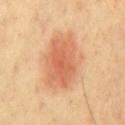No biopsy was performed on this lesion — it was imaged during a full skin examination and was not determined to be concerning.
About 7 mm across.
The tile uses cross-polarized illumination.
A 15 mm close-up tile from a total-body photography series done for melanoma screening.
The subject is a male approximately 70 years of age.
On the chest.